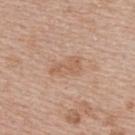Imaged during a routine full-body skin examination; the lesion was not biopsied and no histopathology is available. A 15 mm close-up extracted from a 3D total-body photography capture. The lesion is on the back. An algorithmic analysis of the crop reported a lesion area of about 7.5 mm² and a symmetry-axis asymmetry near 0.35. It also reported a border-irregularity index near 3.5/10 and a peripheral color-asymmetry measure near 0.5. It also reported a detector confidence of about 100 out of 100 that the crop contains a lesion. Approximately 4 mm at its widest. The subject is a female roughly 50 years of age.